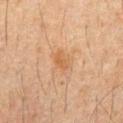Clinical impression:
Recorded during total-body skin imaging; not selected for excision or biopsy.
Image and clinical context:
Approximately 3 mm at its widest. A roughly 15 mm field-of-view crop from a total-body skin photograph. The lesion is on the chest. A male patient, aged approximately 60.